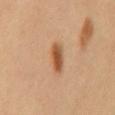image source = ~15 mm crop, total-body skin-cancer survey
subject = female, approximately 50 years of age